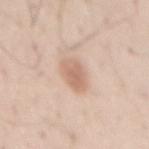biopsy_status: not biopsied; imaged during a skin examination
site: lower back
automated_metrics:
  border_irregularity_0_10: 1.5
  color_variation_0_10: 2.5
  peripheral_color_asymmetry: 1.0
image:
  source: total-body photography crop
  field_of_view_mm: 15
lesion_size:
  long_diameter_mm_approx: 3.5
patient:
  sex: male
  age_approx: 60
lighting: white-light